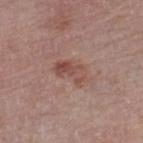This is a white-light tile.
The lesion is on the left thigh.
A roughly 15 mm field-of-view crop from a total-body skin photograph.
The patient is a male about 75 years old.
Approximately 4 mm at its widest.